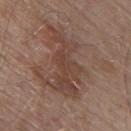  biopsy_status: not biopsied; imaged during a skin examination
  lighting: white-light
  patient:
    sex: male
    age_approx: 55
  image:
    source: total-body photography crop
    field_of_view_mm: 15
  site: upper back
  lesion_size:
    long_diameter_mm_approx: 8.5